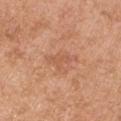biopsy_status: not biopsied; imaged during a skin examination
patient:
  sex: male
  age_approx: 55
image:
  source: total-body photography crop
  field_of_view_mm: 15
site: right upper arm